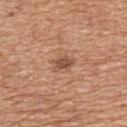Impression: Part of a total-body skin-imaging series; this lesion was reviewed on a skin check and was not flagged for biopsy. Acquisition and patient details: Automated tile analysis of the lesion measured a lesion area of about 3.5 mm², a shape eccentricity near 0.75, and a symmetry-axis asymmetry near 0.3. The software also gave an average lesion color of about L≈51 a*≈22 b*≈31 (CIELAB) and a normalized lesion–skin contrast near 7.5. From the upper back. Imaged with white-light lighting. A close-up tile cropped from a whole-body skin photograph, about 15 mm across. The recorded lesion diameter is about 2.5 mm. A female subject aged approximately 55.